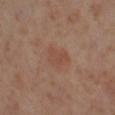Clinical impression:
Recorded during total-body skin imaging; not selected for excision or biopsy.
Context:
Longest diameter approximately 3 mm. This image is a 15 mm lesion crop taken from a total-body photograph. A female subject, aged approximately 55. On the leg. The tile uses cross-polarized illumination. The lesion-visualizer software estimated a nevus-likeness score of about 25/100.